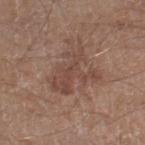Assessment:
Part of a total-body skin-imaging series; this lesion was reviewed on a skin check and was not flagged for biopsy.
Acquisition and patient details:
Automated tile analysis of the lesion measured a footprint of about 14 mm², a shape eccentricity near 0.7, and a symmetry-axis asymmetry near 0.55. And it measured a classifier nevus-likeness of about 0/100 and lesion-presence confidence of about 100/100. The lesion is located on the right lower leg. A lesion tile, about 15 mm wide, cut from a 3D total-body photograph. The patient is a male aged 58–62.